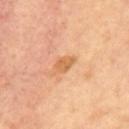Assessment: The lesion was tiled from a total-body skin photograph and was not biopsied. Clinical summary: Approximately 2.5 mm at its widest. A 15 mm close-up extracted from a 3D total-body photography capture. The tile uses cross-polarized illumination. From the mid back. The patient is a female in their mid- to late 40s.